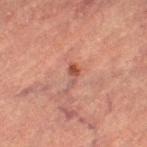Impression: Captured during whole-body skin photography for melanoma surveillance; the lesion was not biopsied. Image and clinical context: Cropped from a whole-body photographic skin survey; the tile spans about 15 mm. The tile uses cross-polarized illumination. A female subject, aged 68 to 72. The lesion is on the leg.